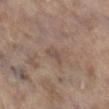Q: Was this lesion biopsied?
A: no biopsy performed (imaged during a skin exam)
Q: What are the patient's age and sex?
A: female, in their mid- to late 80s or older
Q: What is the imaging modality?
A: ~15 mm crop, total-body skin-cancer survey
Q: Where on the body is the lesion?
A: the right lower leg